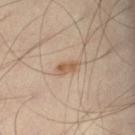No biopsy was performed on this lesion — it was imaged during a full skin examination and was not determined to be concerning.
A 15 mm close-up extracted from a 3D total-body photography capture.
A male subject approximately 35 years of age.
On the right leg.